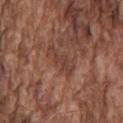Q: Was this lesion biopsied?
A: no biopsy performed (imaged during a skin exam)
Q: What is the anatomic site?
A: the chest
Q: What lighting was used for the tile?
A: white-light
Q: How was this image acquired?
A: ~15 mm tile from a whole-body skin photo
Q: What is the lesion's diameter?
A: about 4 mm
Q: Patient demographics?
A: male, approximately 75 years of age
Q: What did automated image analysis measure?
A: an automated nevus-likeness rating near 0 out of 100